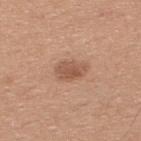{
  "automated_metrics": {
    "area_mm2_approx": 6.5,
    "eccentricity": 0.8,
    "shape_asymmetry": 0.2,
    "cielab_L": 54,
    "cielab_a": 21,
    "cielab_b": 30,
    "vs_skin_darker_L": 10.0
  },
  "patient": {
    "sex": "male",
    "age_approx": 30
  },
  "site": "upper back",
  "image": {
    "source": "total-body photography crop",
    "field_of_view_mm": 15
  }
}